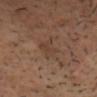Impression:
Part of a total-body skin-imaging series; this lesion was reviewed on a skin check and was not flagged for biopsy.
Background:
Measured at roughly 3.5 mm in maximum diameter. A 15 mm crop from a total-body photograph taken for skin-cancer surveillance. Imaged with cross-polarized lighting. The subject is a male approximately 40 years of age. The total-body-photography lesion software estimated a lesion area of about 5.5 mm², a shape eccentricity near 0.8, and a symmetry-axis asymmetry near 0.3. The software also gave a lesion color around L≈38 a*≈16 b*≈26 in CIELAB, a lesion–skin lightness drop of about 5, and a normalized border contrast of about 5. The analysis additionally found internal color variation of about 2 on a 0–10 scale and radial color variation of about 0.5. The lesion is located on the head or neck.